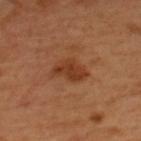Findings:
- notes — total-body-photography surveillance lesion; no biopsy
- patient — male, aged approximately 50
- imaging modality — total-body-photography crop, ~15 mm field of view
- anatomic site — the upper back